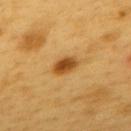Clinical summary:
The lesion is on the upper back. Captured under cross-polarized illumination. A male patient, aged 58–62. Automated tile analysis of the lesion measured a footprint of about 5 mm², a shape eccentricity near 0.8, and a shape-asymmetry score of about 0.15 (0 = symmetric). It also reported a classifier nevus-likeness of about 100/100 and lesion-presence confidence of about 100/100. Cropped from a total-body skin-imaging series; the visible field is about 15 mm.